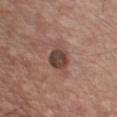Q: Lesion location?
A: the chest
Q: Who is the patient?
A: male, in their mid-50s
Q: What is the imaging modality?
A: ~15 mm tile from a whole-body skin photo
Q: What did automated image analysis measure?
A: a footprint of about 6.5 mm², an eccentricity of roughly 0.25, and a symmetry-axis asymmetry near 0.2; a mean CIELAB color near L≈42 a*≈18 b*≈22, a lesion–skin lightness drop of about 13, and a lesion-to-skin contrast of about 10 (normalized; higher = more distinct); peripheral color asymmetry of about 1
Q: How was the tile lit?
A: white-light illumination